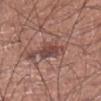Recorded during total-body skin imaging; not selected for excision or biopsy.
A 15 mm close-up extracted from a 3D total-body photography capture.
Located on the right lower leg.
The subject is a male aged approximately 45.